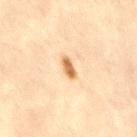<lesion>
  <site>right thigh</site>
  <image>
    <source>total-body photography crop</source>
    <field_of_view_mm>15</field_of_view_mm>
  </image>
  <patient>
    <sex>female</sex>
    <age_approx>45</age_approx>
  </patient>
  <automated_metrics>
    <shape_asymmetry>0.25</shape_asymmetry>
    <cielab_L>60</cielab_L>
    <cielab_a>19</cielab_a>
    <cielab_b>38</cielab_b>
    <vs_skin_darker_L>14.0</vs_skin_darker_L>
    <vs_skin_contrast_norm>9.5</vs_skin_contrast_norm>
  </automated_metrics>
</lesion>